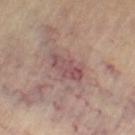Findings:
• biopsy status: catalogued during a skin exam; not biopsied
• location: the left thigh
• tile lighting: cross-polarized
• lesion size: about 4 mm
• subject: female, in their mid-60s
• image source: ~15 mm tile from a whole-body skin photo